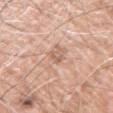Imaged during a routine full-body skin examination; the lesion was not biopsied and no histopathology is available.
The subject is a male aged 58–62.
The lesion-visualizer software estimated an area of roughly 2.5 mm², a shape eccentricity near 0.9, and a symmetry-axis asymmetry near 0.35. The software also gave roughly 9 lightness units darker than nearby skin. And it measured a peripheral color-asymmetry measure near 0. And it measured an automated nevus-likeness rating near 0 out of 100.
The recorded lesion diameter is about 2.5 mm.
This image is a 15 mm lesion crop taken from a total-body photograph.
The lesion is on the right upper arm.
The tile uses white-light illumination.